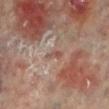• biopsy status · catalogued during a skin exam; not biopsied
• subject · female, in their 60s
• site · the left lower leg
• image source · total-body-photography crop, ~15 mm field of view